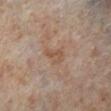The lesion was photographed on a routine skin check and not biopsied; there is no pathology result. Automated image analysis of the tile measured a lesion area of about 4.5 mm² and an eccentricity of roughly 0.8. The software also gave border irregularity of about 4.5 on a 0–10 scale, internal color variation of about 2.5 on a 0–10 scale, and radial color variation of about 1. The software also gave an automated nevus-likeness rating near 0 out of 100. Captured under cross-polarized illumination. Approximately 3 mm at its widest. A female patient, aged approximately 50. Located on the leg. A roughly 15 mm field-of-view crop from a total-body skin photograph.